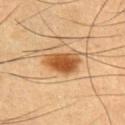Notes:
• notes: no biopsy performed (imaged during a skin exam)
• imaging modality: total-body-photography crop, ~15 mm field of view
• patient: male, aged around 55
• tile lighting: cross-polarized
• automated metrics: an average lesion color of about L≈46 a*≈21 b*≈37 (CIELAB), roughly 14 lightness units darker than nearby skin, and a normalized border contrast of about 10.5; a border-irregularity rating of about 2.5/10 and radial color variation of about 1
• anatomic site: the upper back
• lesion size: ~5 mm (longest diameter)